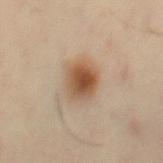The lesion is on the right thigh.
A female subject approximately 45 years of age.
A region of skin cropped from a whole-body photographic capture, roughly 15 mm wide.
Longest diameter approximately 3.5 mm.
Captured under cross-polarized illumination.